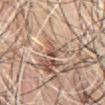| field | value |
|---|---|
| workup | no biopsy performed (imaged during a skin exam) |
| patient | male, roughly 65 years of age |
| automated metrics | a shape-asymmetry score of about 0.35 (0 = symmetric); a lesion color around L≈49 a*≈19 b*≈27 in CIELAB |
| lesion diameter | ~2 mm (longest diameter) |
| image source | ~15 mm tile from a whole-body skin photo |
| location | the front of the torso |